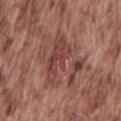<case>
<biopsy_status>not biopsied; imaged during a skin examination</biopsy_status>
<image>
  <source>total-body photography crop</source>
  <field_of_view_mm>15</field_of_view_mm>
</image>
<patient>
  <sex>male</sex>
  <age_approx>75</age_approx>
</patient>
<site>mid back</site>
</case>